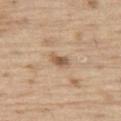<record>
<biopsy_status>not biopsied; imaged during a skin examination</biopsy_status>
<patient>
  <sex>male</sex>
  <age_approx>70</age_approx>
</patient>
<site>left thigh</site>
<lesion_size>
  <long_diameter_mm_approx>2.5</long_diameter_mm_approx>
</lesion_size>
<lighting>white-light</lighting>
<image>
  <source>total-body photography crop</source>
  <field_of_view_mm>15</field_of_view_mm>
</image>
<automated_metrics>
  <vs_skin_darker_L>11.0</vs_skin_darker_L>
  <vs_skin_contrast_norm>7.5</vs_skin_contrast_norm>
  <border_irregularity_0_10>2.5</border_irregularity_0_10>
  <color_variation_0_10>3.5</color_variation_0_10>
  <peripheral_color_asymmetry>1.5</peripheral_color_asymmetry>
</automated_metrics>
</record>